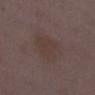<lesion>
  <biopsy_status>not biopsied; imaged during a skin examination</biopsy_status>
  <automated_metrics>
    <area_mm2_approx>15.0</area_mm2_approx>
    <eccentricity>0.8</eccentricity>
    <shape_asymmetry>0.2</shape_asymmetry>
    <cielab_L>37</cielab_L>
    <cielab_a>14</cielab_a>
    <cielab_b>18</cielab_b>
  </automated_metrics>
  <image>
    <source>total-body photography crop</source>
    <field_of_view_mm>15</field_of_view_mm>
  </image>
  <site>right lower leg</site>
  <patient>
    <sex>female</sex>
    <age_approx>30</age_approx>
  </patient>
  <lesion_size>
    <long_diameter_mm_approx>5.5</long_diameter_mm_approx>
  </lesion_size>
  <lighting>white-light</lighting>
</lesion>